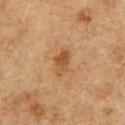Clinical impression: Part of a total-body skin-imaging series; this lesion was reviewed on a skin check and was not flagged for biopsy. Context: The tile uses cross-polarized illumination. From the chest. Automated image analysis of the tile measured a lesion area of about 5.5 mm², a shape eccentricity near 0.8, and a symmetry-axis asymmetry near 0.2. It also reported about 8 CIELAB-L* units darker than the surrounding skin and a normalized border contrast of about 7. And it measured border irregularity of about 2.5 on a 0–10 scale, internal color variation of about 4 on a 0–10 scale, and radial color variation of about 1.5. It also reported a nevus-likeness score of about 65/100 and lesion-presence confidence of about 100/100. A male patient about 75 years old. The lesion's longest dimension is about 3.5 mm. Cropped from a total-body skin-imaging series; the visible field is about 15 mm.